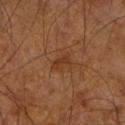  biopsy_status: not biopsied; imaged during a skin examination
  image:
    source: total-body photography crop
    field_of_view_mm: 15
  patient:
    sex: male
    age_approx: 65
  automated_metrics:
    area_mm2_approx: 3.5
    eccentricity: 0.7
    shape_asymmetry: 0.3
    cielab_L: 36
    cielab_a: 22
    cielab_b: 33
    vs_skin_contrast_norm: 5.5
  site: right upper arm
  lesion_size:
    long_diameter_mm_approx: 2.5
  lighting: cross-polarized